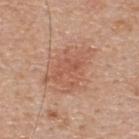No biopsy was performed on this lesion — it was imaged during a full skin examination and was not determined to be concerning. The lesion is on the upper back. The tile uses white-light illumination. A male patient, aged 53 to 57. Automated image analysis of the tile measured an area of roughly 22 mm². It also reported a mean CIELAB color near L≈57 a*≈22 b*≈31 and about 7 CIELAB-L* units darker than the surrounding skin. It also reported border irregularity of about 4 on a 0–10 scale and a color-variation rating of about 3.5/10. The analysis additionally found a nevus-likeness score of about 50/100 and lesion-presence confidence of about 100/100. Cropped from a whole-body photographic skin survey; the tile spans about 15 mm. Approximately 6 mm at its widest.